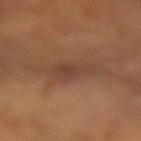Captured during whole-body skin photography for melanoma surveillance; the lesion was not biopsied.
A female subject roughly 55 years of age.
The lesion is on the right forearm.
A roughly 15 mm field-of-view crop from a total-body skin photograph.
The recorded lesion diameter is about 6.5 mm.
The tile uses cross-polarized illumination.